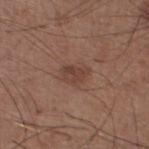Recorded during total-body skin imaging; not selected for excision or biopsy. About 3 mm across. On the left lower leg. Automated image analysis of the tile measured a footprint of about 5 mm² and an outline eccentricity of about 0.75 (0 = round, 1 = elongated). The software also gave a nevus-likeness score of about 20/100 and a lesion-detection confidence of about 100/100. This image is a 15 mm lesion crop taken from a total-body photograph. The subject is a male in their 60s. Captured under white-light illumination.